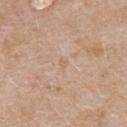biopsy status: total-body-photography surveillance lesion; no biopsy | acquisition: 15 mm crop, total-body photography | subject: female, about 60 years old | tile lighting: white-light illumination | lesion diameter: ≈1.5 mm | anatomic site: the upper back.